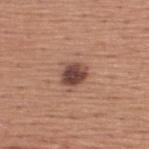Image and clinical context: From the upper back. A close-up tile cropped from a whole-body skin photograph, about 15 mm across. A male subject, approximately 45 years of age.